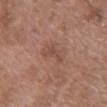| field | value |
|---|---|
| follow-up | imaged on a skin check; not biopsied |
| acquisition | 15 mm crop, total-body photography |
| lesion diameter | ≈3.5 mm |
| automated lesion analysis | a nevus-likeness score of about 0/100 and a detector confidence of about 100 out of 100 that the crop contains a lesion |
| lighting | white-light illumination |
| patient | female, aged 68 to 72 |
| site | the chest |